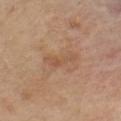<case>
<biopsy_status>not biopsied; imaged during a skin examination</biopsy_status>
<patient>
  <sex>male</sex>
  <age_approx>70</age_approx>
</patient>
<lesion_size>
  <long_diameter_mm_approx>3.5</long_diameter_mm_approx>
</lesion_size>
<automated_metrics>
  <border_irregularity_0_10>7.0</border_irregularity_0_10>
  <peripheral_color_asymmetry>0.0</peripheral_color_asymmetry>
</automated_metrics>
<site>chest</site>
<lighting>cross-polarized</lighting>
<image>
  <source>total-body photography crop</source>
  <field_of_view_mm>15</field_of_view_mm>
</image>
</case>